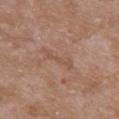The lesion is located on the right upper arm. Imaged with white-light lighting. Measured at roughly 4 mm in maximum diameter. A 15 mm close-up extracted from a 3D total-body photography capture. A female subject roughly 70 years of age. Automated image analysis of the tile measured an average lesion color of about L≈51 a*≈19 b*≈29 (CIELAB), roughly 6 lightness units darker than nearby skin, and a normalized border contrast of about 4.5.